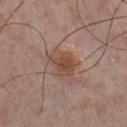Assessment:
No biopsy was performed on this lesion — it was imaged during a full skin examination and was not determined to be concerning.
Background:
The lesion is on the chest. A 15 mm close-up tile from a total-body photography series done for melanoma screening. Imaged with white-light lighting. An algorithmic analysis of the crop reported a lesion area of about 8 mm², an outline eccentricity of about 0.65 (0 = round, 1 = elongated), and a symmetry-axis asymmetry near 0.25. And it measured a lesion–skin lightness drop of about 9. And it measured border irregularity of about 2.5 on a 0–10 scale and a peripheral color-asymmetry measure near 1.5. It also reported a nevus-likeness score of about 95/100 and a detector confidence of about 100 out of 100 that the crop contains a lesion. The recorded lesion diameter is about 3.5 mm. A male subject about 55 years old.